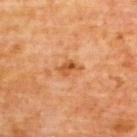From the upper back. Captured under cross-polarized illumination. The patient is a male aged approximately 60. A 15 mm close-up tile from a total-body photography series done for melanoma screening. About 2.5 mm across.